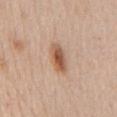notes: total-body-photography surveillance lesion; no biopsy.